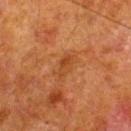{
  "biopsy_status": "not biopsied; imaged during a skin examination",
  "patient": {
    "sex": "male",
    "age_approx": 80
  },
  "site": "right lower leg",
  "image": {
    "source": "total-body photography crop",
    "field_of_view_mm": 15
  }
}